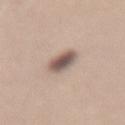Clinical impression:
No biopsy was performed on this lesion — it was imaged during a full skin examination and was not determined to be concerning.
Image and clinical context:
Automated tile analysis of the lesion measured an average lesion color of about L≈55 a*≈14 b*≈22 (CIELAB) and a normalized border contrast of about 10. It also reported border irregularity of about 1 on a 0–10 scale and a color-variation rating of about 6/10. Cropped from a total-body skin-imaging series; the visible field is about 15 mm. On the lower back. The subject is a female aged around 30. Approximately 3 mm at its widest.